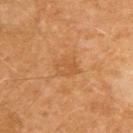Imaged during a routine full-body skin examination; the lesion was not biopsied and no histopathology is available.
Approximately 3.5 mm at its widest.
A region of skin cropped from a whole-body photographic capture, roughly 15 mm wide.
The subject is a male approximately 60 years of age.
The lesion is located on the upper back.